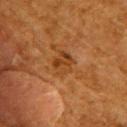  biopsy_status: not biopsied; imaged during a skin examination
  site: back
  patient:
    sex: female
    age_approx: 50
  lesion_size:
    long_diameter_mm_approx: 3.0
  image:
    source: total-body photography crop
    field_of_view_mm: 15
  lighting: cross-polarized